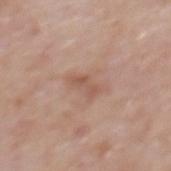Q: Was this lesion biopsied?
A: no biopsy performed (imaged during a skin exam)
Q: What kind of image is this?
A: 15 mm crop, total-body photography
Q: What are the patient's age and sex?
A: male, aged approximately 75
Q: How large is the lesion?
A: ~3 mm (longest diameter)
Q: What did automated image analysis measure?
A: a mean CIELAB color near L≈54 a*≈21 b*≈28, roughly 8 lightness units darker than nearby skin, and a normalized border contrast of about 6
Q: Lesion location?
A: the mid back
Q: Illumination type?
A: white-light